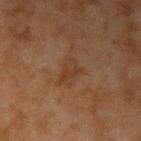A region of skin cropped from a whole-body photographic capture, roughly 15 mm wide. A male patient in their mid- to late 40s. Captured under cross-polarized illumination. Automated tile analysis of the lesion measured a border-irregularity index near 5.5/10, internal color variation of about 1.5 on a 0–10 scale, and radial color variation of about 0.5. It also reported a lesion-detection confidence of about 100/100. The lesion's longest dimension is about 3 mm. The lesion is on the right upper arm.